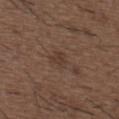<case>
  <biopsy_status>not biopsied; imaged during a skin examination</biopsy_status>
  <image>
    <source>total-body photography crop</source>
    <field_of_view_mm>15</field_of_view_mm>
  </image>
  <automated_metrics>
    <area_mm2_approx>3.0</area_mm2_approx>
    <eccentricity>0.75</eccentricity>
    <shape_asymmetry>0.45</shape_asymmetry>
    <cielab_L>36</cielab_L>
    <cielab_a>16</cielab_a>
    <cielab_b>23</cielab_b>
    <vs_skin_darker_L>6.0</vs_skin_darker_L>
    <vs_skin_contrast_norm>6.0</vs_skin_contrast_norm>
    <border_irregularity_0_10>4.5</border_irregularity_0_10>
    <color_variation_0_10>1.0</color_variation_0_10>
    <peripheral_color_asymmetry>0.5</peripheral_color_asymmetry>
    <nevus_likeness_0_100>0</nevus_likeness_0_100>
    <lesion_detection_confidence_0_100>100</lesion_detection_confidence_0_100>
  </automated_metrics>
  <patient>
    <sex>male</sex>
    <age_approx>50</age_approx>
  </patient>
  <lesion_size>
    <long_diameter_mm_approx>2.5</long_diameter_mm_approx>
  </lesion_size>
  <site>upper back</site>
  <lighting>white-light</lighting>
</case>